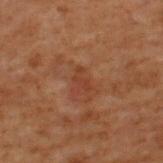<lesion>
  <biopsy_status>not biopsied; imaged during a skin examination</biopsy_status>
  <site>upper back</site>
  <image>
    <source>total-body photography crop</source>
    <field_of_view_mm>15</field_of_view_mm>
  </image>
  <patient>
    <sex>male</sex>
    <age_approx>70</age_approx>
  </patient>
  <lesion_size>
    <long_diameter_mm_approx>2.5</long_diameter_mm_approx>
  </lesion_size>
  <lighting>cross-polarized</lighting>
</lesion>